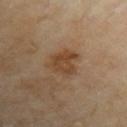Part of a total-body skin-imaging series; this lesion was reviewed on a skin check and was not flagged for biopsy. A 15 mm crop from a total-body photograph taken for skin-cancer surveillance. The lesion is located on the left upper arm. Captured under cross-polarized illumination. A male subject, aged 68–72. The recorded lesion diameter is about 4 mm.